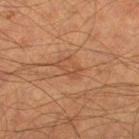Assessment: The lesion was photographed on a routine skin check and not biopsied; there is no pathology result. Context: A region of skin cropped from a whole-body photographic capture, roughly 15 mm wide. A male subject aged 63–67. From the right thigh.